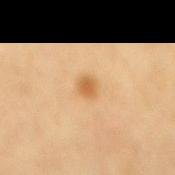The lesion was photographed on a routine skin check and not biopsied; there is no pathology result.
A region of skin cropped from a whole-body photographic capture, roughly 15 mm wide.
A female patient in their 70s.
The lesion is on the mid back.
About 2 mm across.